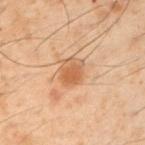| field | value |
|---|---|
| workup | total-body-photography surveillance lesion; no biopsy |
| body site | the left upper arm |
| acquisition | ~15 mm crop, total-body skin-cancer survey |
| TBP lesion metrics | a footprint of about 8 mm², a shape eccentricity near 0.55, and two-axis asymmetry of about 0.2; a mean CIELAB color near L≈46 a*≈18 b*≈30 and roughly 8 lightness units darker than nearby skin; a classifier nevus-likeness of about 85/100 and a detector confidence of about 100 out of 100 that the crop contains a lesion |
| patient | male, aged 48 to 52 |
| lesion size | ≈3.5 mm |
| lighting | cross-polarized |